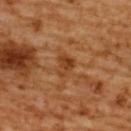workup — total-body-photography surveillance lesion; no biopsy
image — 15 mm crop, total-body photography
lighting — cross-polarized illumination
body site — the upper back
subject — female, aged around 55
size — ≈3 mm